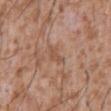Clinical impression: Recorded during total-body skin imaging; not selected for excision or biopsy. Acquisition and patient details: Imaged with white-light lighting. The lesion-visualizer software estimated an average lesion color of about L≈52 a*≈20 b*≈30 (CIELAB) and a lesion–skin lightness drop of about 7. The software also gave internal color variation of about 0.5 on a 0–10 scale and radial color variation of about 0.5. Located on the abdomen. A lesion tile, about 15 mm wide, cut from a 3D total-body photograph. The recorded lesion diameter is about 2.5 mm. A male patient aged approximately 45.